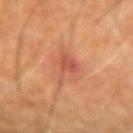workup = no biopsy performed (imaged during a skin exam) | acquisition = 15 mm crop, total-body photography | subject = male, aged around 60 | illumination = cross-polarized | location = the arm | TBP lesion metrics = a footprint of about 5.5 mm² and two-axis asymmetry of about 0.5; a lesion color around L≈51 a*≈28 b*≈32 in CIELAB and a normalized lesion–skin contrast near 6; a border-irregularity rating of about 6.5/10 and internal color variation of about 4.5 on a 0–10 scale; a classifier nevus-likeness of about 0/100 and a lesion-detection confidence of about 100/100 | lesion diameter = about 4.5 mm.